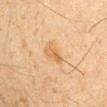follow-up — catalogued during a skin exam; not biopsied
TBP lesion metrics — a footprint of about 4.5 mm², an outline eccentricity of about 0.9 (0 = round, 1 = elongated), and a shape-asymmetry score of about 0.4 (0 = symmetric)
anatomic site — the right upper arm
subject — male, roughly 35 years of age
imaging modality — total-body-photography crop, ~15 mm field of view
illumination — cross-polarized illumination